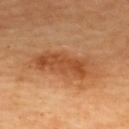Assessment: This lesion was catalogued during total-body skin photography and was not selected for biopsy. Image and clinical context: The tile uses cross-polarized illumination. The total-body-photography lesion software estimated a lesion color around L≈52 a*≈26 b*≈41 in CIELAB and a normalized border contrast of about 7.5. Located on the upper back. A 15 mm close-up extracted from a 3D total-body photography capture. A female subject, in their 60s.